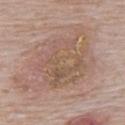biopsy status: imaged on a skin check; not biopsied
image: 15 mm crop, total-body photography
automated metrics: a footprint of about 30 mm², an outline eccentricity of about 0.4 (0 = round, 1 = elongated), and two-axis asymmetry of about 0.25; an average lesion color of about L≈55 a*≈16 b*≈27 (CIELAB) and about 6 CIELAB-L* units darker than the surrounding skin; an automated nevus-likeness rating near 0 out of 100 and a lesion-detection confidence of about 95/100
illumination: white-light
patient: male, in their mid-70s
size: ≈7 mm
body site: the upper back